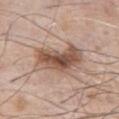Findings:
* workup — no biopsy performed (imaged during a skin exam)
* image — 15 mm crop, total-body photography
* patient — male, approximately 80 years of age
* lighting — white-light
* site — the chest